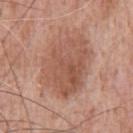Captured during whole-body skin photography for melanoma surveillance; the lesion was not biopsied.
From the front of the torso.
Measured at roughly 8 mm in maximum diameter.
This is a white-light tile.
A region of skin cropped from a whole-body photographic capture, roughly 15 mm wide.
The subject is a male roughly 60 years of age.